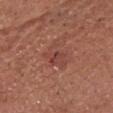Background: A lesion tile, about 15 mm wide, cut from a 3D total-body photograph. This is a white-light tile. On the chest. The lesion's longest dimension is about 3.5 mm. A male patient, about 75 years old.